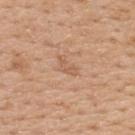No biopsy was performed on this lesion — it was imaged during a full skin examination and was not determined to be concerning.
Cropped from a total-body skin-imaging series; the visible field is about 15 mm.
Imaged with white-light lighting.
The lesion is on the upper back.
Measured at roughly 3 mm in maximum diameter.
The lesion-visualizer software estimated an eccentricity of roughly 0.9 and a shape-asymmetry score of about 0.5 (0 = symmetric). The analysis additionally found a detector confidence of about 100 out of 100 that the crop contains a lesion.
A female subject, aged approximately 45.